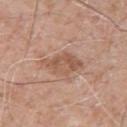Q: Was this lesion biopsied?
A: catalogued during a skin exam; not biopsied
Q: How large is the lesion?
A: about 4.5 mm
Q: How was this image acquired?
A: ~15 mm tile from a whole-body skin photo
Q: What did automated image analysis measure?
A: a lesion color around L≈55 a*≈20 b*≈29 in CIELAB, about 9 CIELAB-L* units darker than the surrounding skin, and a normalized lesion–skin contrast near 6.5; a border-irregularity index near 3.5/10 and a color-variation rating of about 3.5/10
Q: What is the anatomic site?
A: the right upper arm
Q: What lighting was used for the tile?
A: white-light illumination
Q: Patient demographics?
A: male, aged approximately 60